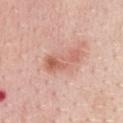The lesion was tiled from a total-body skin photograph and was not biopsied. Automated image analysis of the tile measured an area of roughly 8 mm², a shape eccentricity near 0.9, and two-axis asymmetry of about 0.35. The software also gave a border-irregularity rating of about 4.5/10. The software also gave a classifier nevus-likeness of about 20/100 and lesion-presence confidence of about 100/100. A female subject, aged around 40. The recorded lesion diameter is about 5 mm. A 15 mm close-up tile from a total-body photography series done for melanoma screening. The lesion is located on the chest.